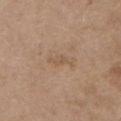workup: total-body-photography surveillance lesion; no biopsy | TBP lesion metrics: a lesion color around L≈54 a*≈16 b*≈31 in CIELAB and a lesion–skin lightness drop of about 6; a color-variation rating of about 0/10 and a peripheral color-asymmetry measure near 0; lesion-presence confidence of about 100/100 | patient: female, approximately 65 years of age | diameter: ≈3 mm | location: the chest | image: ~15 mm crop, total-body skin-cancer survey | lighting: white-light.